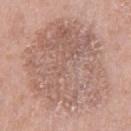<case>
  <lesion_size>
    <long_diameter_mm_approx>11.0</long_diameter_mm_approx>
  </lesion_size>
  <site>left thigh</site>
  <patient>
    <sex>female</sex>
    <age_approx>55</age_approx>
  </patient>
  <automated_metrics>
    <area_mm2_approx>75.0</area_mm2_approx>
    <cielab_L>60</cielab_L>
    <cielab_a>18</cielab_a>
    <cielab_b>25</cielab_b>
    <vs_skin_darker_L>8.0</vs_skin_darker_L>
  </automated_metrics>
  <image>
    <source>total-body photography crop</source>
    <field_of_view_mm>15</field_of_view_mm>
  </image>
</case>